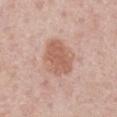Case summary:
- workup: total-body-photography surveillance lesion; no biopsy
- illumination: white-light
- patient: male, roughly 60 years of age
- size: about 5 mm
- imaging modality: total-body-photography crop, ~15 mm field of view
- location: the right upper arm